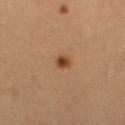Assessment: Imaged during a routine full-body skin examination; the lesion was not biopsied and no histopathology is available. Clinical summary: The lesion is located on the right thigh. Cropped from a total-body skin-imaging series; the visible field is about 15 mm. The subject is a female approximately 55 years of age.